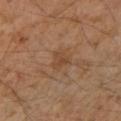The lesion was tiled from a total-body skin photograph and was not biopsied. The lesion-visualizer software estimated a mean CIELAB color near L≈45 a*≈19 b*≈32 and a normalized border contrast of about 5.5. The software also gave border irregularity of about 3.5 on a 0–10 scale, internal color variation of about 2 on a 0–10 scale, and peripheral color asymmetry of about 0.5. It also reported a classifier nevus-likeness of about 0/100. The tile uses cross-polarized illumination. Located on the arm. A 15 mm crop from a total-body photograph taken for skin-cancer surveillance. A female patient, in their mid- to late 40s. About 2.5 mm across.